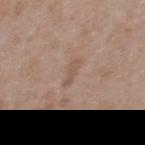follow-up = catalogued during a skin exam; not biopsied | tile lighting = white-light | location = the upper back | imaging modality = 15 mm crop, total-body photography | size = ~2.5 mm (longest diameter) | patient = female, about 30 years old | automated metrics = a lesion area of about 2.5 mm², a shape eccentricity near 0.9, and two-axis asymmetry of about 0.3; a color-variation rating of about 0/10; a nevus-likeness score of about 0/100 and a detector confidence of about 100 out of 100 that the crop contains a lesion.